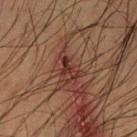The lesion was photographed on a routine skin check and not biopsied; there is no pathology result.